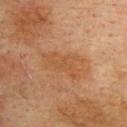This lesion was catalogued during total-body skin photography and was not selected for biopsy. Cropped from a total-body skin-imaging series; the visible field is about 15 mm. Imaged with cross-polarized lighting. Located on the upper back. Measured at roughly 5 mm in maximum diameter. A male subject, in their mid-70s. Automated tile analysis of the lesion measured an eccentricity of roughly 0.85 and a symmetry-axis asymmetry near 0.4. And it measured a lesion-detection confidence of about 100/100.